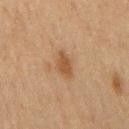This lesion was catalogued during total-body skin photography and was not selected for biopsy. About 3.5 mm across. A male subject aged around 75. A 15 mm close-up extracted from a 3D total-body photography capture. Located on the mid back. Imaged with cross-polarized lighting.